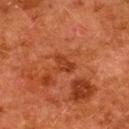{
  "biopsy_status": "not biopsied; imaged during a skin examination",
  "automated_metrics": {
    "shape_asymmetry": 0.35,
    "cielab_L": 33,
    "cielab_a": 27,
    "cielab_b": 33,
    "vs_skin_darker_L": 7.0,
    "vs_skin_contrast_norm": 6.5,
    "border_irregularity_0_10": 3.5,
    "color_variation_0_10": 2.0,
    "peripheral_color_asymmetry": 0.5,
    "nevus_likeness_0_100": 0,
    "lesion_detection_confidence_0_100": 100
  },
  "lighting": "cross-polarized",
  "patient": {
    "sex": "female",
    "age_approx": 50
  },
  "image": {
    "source": "total-body photography crop",
    "field_of_view_mm": 15
  },
  "site": "upper back"
}